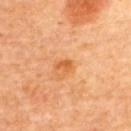| key | value |
|---|---|
| automated metrics | a lesion area of about 1.5 mm², an outline eccentricity of about 0.8 (0 = round, 1 = elongated), and a shape-asymmetry score of about 0.45 (0 = symmetric); a lesion color around L≈58 a*≈30 b*≈47 in CIELAB and about 10 CIELAB-L* units darker than the surrounding skin |
| subject | female, approximately 65 years of age |
| diameter | ~2 mm (longest diameter) |
| image source | ~15 mm crop, total-body skin-cancer survey |
| anatomic site | the back |